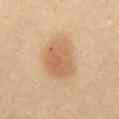<tbp_lesion>
<biopsy_status>not biopsied; imaged during a skin examination</biopsy_status>
<site>abdomen</site>
<patient>
  <sex>female</sex>
  <age_approx>60</age_approx>
</patient>
<lesion_size>
  <long_diameter_mm_approx>5.0</long_diameter_mm_approx>
</lesion_size>
<lighting>cross-polarized</lighting>
<image>
  <source>total-body photography crop</source>
  <field_of_view_mm>15</field_of_view_mm>
</image>
</tbp_lesion>